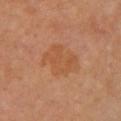No biopsy was performed on this lesion — it was imaged during a full skin examination and was not determined to be concerning. Imaged with cross-polarized lighting. The lesion is on the front of the torso. The recorded lesion diameter is about 5 mm. A 15 mm crop from a total-body photograph taken for skin-cancer surveillance. The patient is a female aged approximately 65.